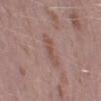Q: Was a biopsy performed?
A: no biopsy performed (imaged during a skin exam)
Q: Lesion location?
A: the left lower leg
Q: How large is the lesion?
A: about 4 mm
Q: Who is the patient?
A: male, aged approximately 40
Q: Automated lesion metrics?
A: a footprint of about 5.5 mm²; a lesion-to-skin contrast of about 5.5 (normalized; higher = more distinct); a within-lesion color-variation index near 2/10 and peripheral color asymmetry of about 0.5
Q: What kind of image is this?
A: ~15 mm crop, total-body skin-cancer survey